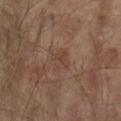biopsy status: total-body-photography surveillance lesion; no biopsy | image source: ~15 mm crop, total-body skin-cancer survey | size: ≈2.5 mm | subject: male, aged approximately 65 | anatomic site: the left forearm.